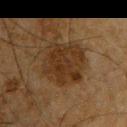{
  "biopsy_status": "not biopsied; imaged during a skin examination",
  "patient": {
    "sex": "male",
    "age_approx": 65
  },
  "site": "left upper arm",
  "image": {
    "source": "total-body photography crop",
    "field_of_view_mm": 15
  },
  "automated_metrics": {
    "cielab_L": 26,
    "cielab_a": 14,
    "cielab_b": 26,
    "vs_skin_darker_L": 7.0,
    "vs_skin_contrast_norm": 8.5,
    "nevus_likeness_0_100": 25,
    "lesion_detection_confidence_0_100": 100
  },
  "lighting": "cross-polarized"
}